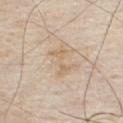No biopsy was performed on this lesion — it was imaged during a full skin examination and was not determined to be concerning.
The tile uses white-light illumination.
A male patient aged around 80.
A roughly 15 mm field-of-view crop from a total-body skin photograph.
The lesion is on the front of the torso.